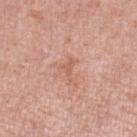  automated_metrics:
    area_mm2_approx: 3.0
    eccentricity: 0.75
    shape_asymmetry: 0.6
    peripheral_color_asymmetry: 0.0
    nevus_likeness_0_100: 0
    lesion_detection_confidence_0_100: 100
  patient:
    sex: female
    age_approx: 40
  image:
    source: total-body photography crop
    field_of_view_mm: 15
  lesion_size:
    long_diameter_mm_approx: 2.5
  lighting: white-light
  site: leg